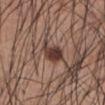Impression:
Recorded during total-body skin imaging; not selected for excision or biopsy.
Image and clinical context:
Longest diameter approximately 3 mm. The lesion is on the front of the torso. An algorithmic analysis of the crop reported an area of roughly 7 mm², an outline eccentricity of about 0.55 (0 = round, 1 = elongated), and a shape-asymmetry score of about 0.35 (0 = symmetric). The tile uses white-light illumination. A male subject, approximately 55 years of age. A 15 mm close-up extracted from a 3D total-body photography capture.